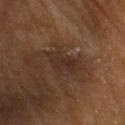notes = no biopsy performed (imaged during a skin exam); subject = male, aged around 85 or older; image = 15 mm crop, total-body photography; body site = the head or neck; size = ≈3.5 mm.